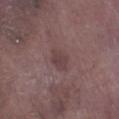workup = total-body-photography surveillance lesion; no biopsy | image = ~15 mm crop, total-body skin-cancer survey | body site = the right lower leg | patient = male, aged 73 to 77 | lesion diameter = ~3 mm (longest diameter) | automated metrics = an area of roughly 4 mm² and two-axis asymmetry of about 0.2; a mean CIELAB color near L≈40 a*≈18 b*≈16 and roughly 7 lightness units darker than nearby skin; a border-irregularity index near 2/10 and peripheral color asymmetry of about 0.5 | lighting = white-light.